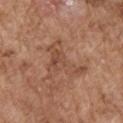The lesion was photographed on a routine skin check and not biopsied; there is no pathology result.
Located on the right upper arm.
This is a white-light tile.
A male patient, in their mid-70s.
The total-body-photography lesion software estimated a lesion–skin lightness drop of about 7. It also reported a nevus-likeness score of about 0/100.
A close-up tile cropped from a whole-body skin photograph, about 15 mm across.